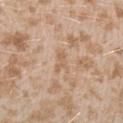<record>
  <biopsy_status>not biopsied; imaged during a skin examination</biopsy_status>
  <patient>
    <sex>female</sex>
    <age_approx>25</age_approx>
  </patient>
  <site>arm</site>
  <automated_metrics>
    <area_mm2_approx>3.0</area_mm2_approx>
    <eccentricity>0.9</eccentricity>
    <cielab_L>61</cielab_L>
    <cielab_a>18</cielab_a>
    <cielab_b>32</cielab_b>
    <vs_skin_darker_L>7.0</vs_skin_darker_L>
    <border_irregularity_0_10>5.5</border_irregularity_0_10>
    <peripheral_color_asymmetry>0.0</peripheral_color_asymmetry>
    <nevus_likeness_0_100>0</nevus_likeness_0_100>
    <lesion_detection_confidence_0_100>70</lesion_detection_confidence_0_100>
  </automated_metrics>
  <lighting>white-light</lighting>
  <image>
    <source>total-body photography crop</source>
    <field_of_view_mm>15</field_of_view_mm>
  </image>
  <lesion_size>
    <long_diameter_mm_approx>3.0</long_diameter_mm_approx>
  </lesion_size>
</record>